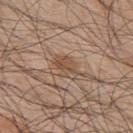Image and clinical context: This is a white-light tile. The recorded lesion diameter is about 3.5 mm. The subject is a male aged around 45. On the upper back. Cropped from a whole-body photographic skin survey; the tile spans about 15 mm.